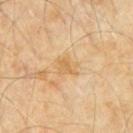Clinical impression:
This lesion was catalogued during total-body skin photography and was not selected for biopsy.
Context:
Measured at roughly 2.5 mm in maximum diameter. This is a cross-polarized tile. Automated image analysis of the tile measured a lesion–skin lightness drop of about 7 and a lesion-to-skin contrast of about 5.5 (normalized; higher = more distinct). The software also gave a classifier nevus-likeness of about 0/100 and a lesion-detection confidence of about 100/100. A male subject about 60 years old. The lesion is located on the chest. A roughly 15 mm field-of-view crop from a total-body skin photograph.